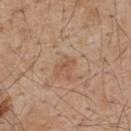The lesion was photographed on a routine skin check and not biopsied; there is no pathology result. A roughly 15 mm field-of-view crop from a total-body skin photograph. The total-body-photography lesion software estimated a lesion color around L≈54 a*≈20 b*≈32 in CIELAB, about 7 CIELAB-L* units darker than the surrounding skin, and a lesion-to-skin contrast of about 5.5 (normalized; higher = more distinct). It also reported a lesion-detection confidence of about 100/100. From the upper back. The subject is a male roughly 55 years of age. This is a white-light tile. Approximately 2.5 mm at its widest.